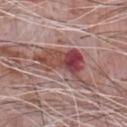follow-up: total-body-photography surveillance lesion; no biopsy | location: the front of the torso | illumination: white-light | diameter: ≈8 mm | subject: male, roughly 70 years of age | image source: 15 mm crop, total-body photography.